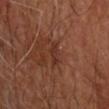Impression: Recorded during total-body skin imaging; not selected for excision or biopsy. Context: The lesion is located on the arm. The patient is a male aged approximately 70. Cropped from a whole-body photographic skin survey; the tile spans about 15 mm. Imaged with cross-polarized lighting.